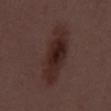- workup: catalogued during a skin exam; not biopsied
- size: ~8.5 mm (longest diameter)
- image-analysis metrics: a footprint of about 21 mm², an outline eccentricity of about 0.95 (0 = round, 1 = elongated), and a shape-asymmetry score of about 0.2 (0 = symmetric); a classifier nevus-likeness of about 80/100
- illumination: white-light illumination
- subject: male, aged 53 to 57
- acquisition: total-body-photography crop, ~15 mm field of view
- anatomic site: the mid back